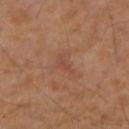<lesion>
<biopsy_status>not biopsied; imaged during a skin examination</biopsy_status>
<lighting>cross-polarized</lighting>
<site>arm</site>
<patient>
  <sex>female</sex>
  <age_approx>60</age_approx>
</patient>
<automated_metrics>
  <cielab_L>48</cielab_L>
  <cielab_a>23</cielab_a>
  <cielab_b>30</cielab_b>
  <vs_skin_darker_L>6.0</vs_skin_darker_L>
  <vs_skin_contrast_norm>5.0</vs_skin_contrast_norm>
  <border_irregularity_0_10>5.5</border_irregularity_0_10>
  <color_variation_0_10>0.0</color_variation_0_10>
  <peripheral_color_asymmetry>0.0</peripheral_color_asymmetry>
  <nevus_likeness_0_100>0</nevus_likeness_0_100>
  <lesion_detection_confidence_0_100>100</lesion_detection_confidence_0_100>
</automated_metrics>
<image>
  <source>total-body photography crop</source>
  <field_of_view_mm>15</field_of_view_mm>
</image>
<lesion_size>
  <long_diameter_mm_approx>3.0</long_diameter_mm_approx>
</lesion_size>
</lesion>